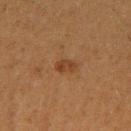subject=female, in their 40s | anatomic site=the right upper arm | lighting=cross-polarized illumination | image source=~15 mm crop, total-body skin-cancer survey.